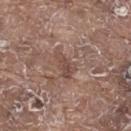This lesion was catalogued during total-body skin photography and was not selected for biopsy.
Cropped from a whole-body photographic skin survey; the tile spans about 15 mm.
Automated image analysis of the tile measured a border-irregularity rating of about 4/10 and radial color variation of about 0.5. The software also gave a classifier nevus-likeness of about 0/100 and a lesion-detection confidence of about 95/100.
On the upper back.
A male subject about 80 years old.